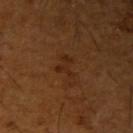  biopsy_status: not biopsied; imaged during a skin examination
  lesion_size:
    long_diameter_mm_approx: 3.0
  site: arm
  lighting: cross-polarized
  patient:
    sex: male
    age_approx: 65
  image:
    source: total-body photography crop
    field_of_view_mm: 15